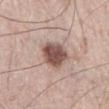* notes — catalogued during a skin exam; not biopsied
* subject — male, aged 68–72
* illumination — white-light
* anatomic site — the right thigh
* image source — ~15 mm tile from a whole-body skin photo
* automated metrics — a lesion area of about 10 mm² and a shape eccentricity near 0.5; a border-irregularity rating of about 1.5/10 and radial color variation of about 1.5